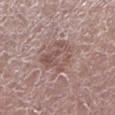Captured during whole-body skin photography for melanoma surveillance; the lesion was not biopsied.
From the left lower leg.
A roughly 15 mm field-of-view crop from a total-body skin photograph.
A male patient aged around 65.
Longest diameter approximately 4.5 mm.